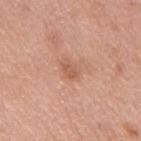Longest diameter approximately 2.5 mm. Automated tile analysis of the lesion measured a mean CIELAB color near L≈58 a*≈24 b*≈32. The analysis additionally found a classifier nevus-likeness of about 0/100 and lesion-presence confidence of about 100/100. Captured under white-light illumination. A roughly 15 mm field-of-view crop from a total-body skin photograph. A male patient roughly 50 years of age. From the mid back.